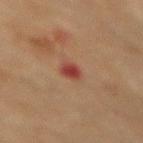This lesion was catalogued during total-body skin photography and was not selected for biopsy. About 2.5 mm across. Located on the mid back. Cropped from a total-body skin-imaging series; the visible field is about 15 mm. The patient is a female aged around 80. The tile uses cross-polarized illumination.